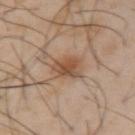Background:
The subject is a male aged approximately 40. This is a cross-polarized tile. On the right upper arm. Automated tile analysis of the lesion measured a symmetry-axis asymmetry near 0.35. And it measured border irregularity of about 3.5 on a 0–10 scale and internal color variation of about 4.5 on a 0–10 scale. The software also gave a classifier nevus-likeness of about 90/100 and a detector confidence of about 100 out of 100 that the crop contains a lesion. Cropped from a total-body skin-imaging series; the visible field is about 15 mm.